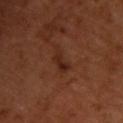Imaged during a routine full-body skin examination; the lesion was not biopsied and no histopathology is available. A male subject aged approximately 50. A lesion tile, about 15 mm wide, cut from a 3D total-body photograph. The lesion is located on the upper back. The tile uses cross-polarized illumination. Automated tile analysis of the lesion measured a shape eccentricity near 0.95 and two-axis asymmetry of about 0.4. The analysis additionally found a normalized lesion–skin contrast near 7. The software also gave an automated nevus-likeness rating near 5 out of 100 and a lesion-detection confidence of about 100/100.